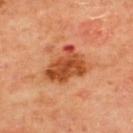  biopsy_status: not biopsied; imaged during a skin examination
  patient:
    sex: male
    age_approx: 65
  automated_metrics:
    border_irregularity_0_10: 4.0
    color_variation_0_10: 5.5
    nevus_likeness_0_100: 10
    lesion_detection_confidence_0_100: 100
  site: upper back
  lighting: cross-polarized
  image:
    source: total-body photography crop
    field_of_view_mm: 15
  lesion_size:
    long_diameter_mm_approx: 5.0